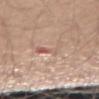The lesion was tiled from a total-body skin photograph and was not biopsied.
This image is a 15 mm lesion crop taken from a total-body photograph.
Located on the left forearm.
A male subject, aged 58–62.